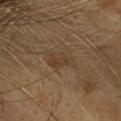Automated image analysis of the tile measured border irregularity of about 3 on a 0–10 scale and a within-lesion color-variation index near 3.5/10. The lesion's longest dimension is about 3.5 mm. A female subject in their 60s. On the head or neck. A 15 mm close-up extracted from a 3D total-body photography capture.